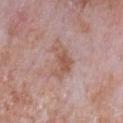Clinical impression: Part of a total-body skin-imaging series; this lesion was reviewed on a skin check and was not flagged for biopsy. Background: Located on the chest. This is a white-light tile. The subject is a male in their mid- to late 60s. The lesion-visualizer software estimated a footprint of about 8 mm², a shape eccentricity near 0.75, and two-axis asymmetry of about 0.5. It also reported a mean CIELAB color near L≈55 a*≈20 b*≈26, roughly 9 lightness units darker than nearby skin, and a normalized lesion–skin contrast near 7. A 15 mm crop from a total-body photograph taken for skin-cancer surveillance.